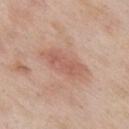<record>
<biopsy_status>not biopsied; imaged during a skin examination</biopsy_status>
<image>
  <source>total-body photography crop</source>
  <field_of_view_mm>15</field_of_view_mm>
</image>
<patient>
  <sex>male</sex>
  <age_approx>55</age_approx>
</patient>
<automated_metrics>
  <area_mm2_approx>13.0</area_mm2_approx>
  <eccentricity>0.9</eccentricity>
  <shape_asymmetry>0.2</shape_asymmetry>
  <cielab_L>59</cielab_L>
  <cielab_a>22</cielab_a>
  <cielab_b>28</cielab_b>
  <vs_skin_darker_L>8.0</vs_skin_darker_L>
  <vs_skin_contrast_norm>5.5</vs_skin_contrast_norm>
  <nevus_likeness_0_100>25</nevus_likeness_0_100>
  <lesion_detection_confidence_0_100>100</lesion_detection_confidence_0_100>
</automated_metrics>
<site>chest</site>
<lesion_size>
  <long_diameter_mm_approx>6.0</long_diameter_mm_approx>
</lesion_size>
<lighting>white-light</lighting>
</record>